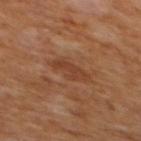workup — catalogued during a skin exam; not biopsied
anatomic site — the back
image-analysis metrics — an area of roughly 7 mm² and a symmetry-axis asymmetry near 0.3; a lesion color around L≈39 a*≈22 b*≈31 in CIELAB and a lesion-to-skin contrast of about 6 (normalized; higher = more distinct); a color-variation rating of about 2/10 and peripheral color asymmetry of about 0.5
subject — male, in their mid-60s
image — ~15 mm crop, total-body skin-cancer survey